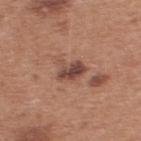Impression:
The lesion was tiled from a total-body skin photograph and was not biopsied.
Context:
The lesion is on the back. A 15 mm crop from a total-body photograph taken for skin-cancer surveillance. A male patient in their mid-50s.